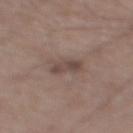The lesion was tiled from a total-body skin photograph and was not biopsied. The tile uses white-light illumination. From the right thigh. A male subject, aged 63 to 67. Approximately 3 mm at its widest. Cropped from a total-body skin-imaging series; the visible field is about 15 mm.